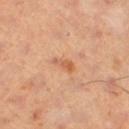No biopsy was performed on this lesion — it was imaged during a full skin examination and was not determined to be concerning. A female patient aged 38–42. Located on the right lower leg. A region of skin cropped from a whole-body photographic capture, roughly 15 mm wide. About 2.5 mm across. This is a cross-polarized tile.